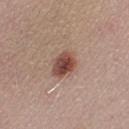The lesion was photographed on a routine skin check and not biopsied; there is no pathology result. This image is a 15 mm lesion crop taken from a total-body photograph. A female patient, aged around 25. Located on the right lower leg.